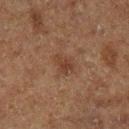{
  "biopsy_status": "not biopsied; imaged during a skin examination",
  "site": "leg",
  "patient": {
    "sex": "male",
    "age_approx": 75
  },
  "lighting": "cross-polarized",
  "image": {
    "source": "total-body photography crop",
    "field_of_view_mm": 15
  },
  "lesion_size": {
    "long_diameter_mm_approx": 2.5
  }
}